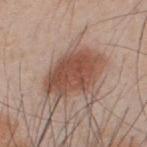Impression:
Part of a total-body skin-imaging series; this lesion was reviewed on a skin check and was not flagged for biopsy.
Context:
Cropped from a total-body skin-imaging series; the visible field is about 15 mm. A male patient aged 33 to 37. From the upper back. The recorded lesion diameter is about 7 mm.